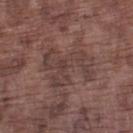Clinical impression: This lesion was catalogued during total-body skin photography and was not selected for biopsy. Image and clinical context: From the left lower leg. The tile uses white-light illumination. The subject is a male aged approximately 75. Longest diameter approximately 6 mm. Cropped from a total-body skin-imaging series; the visible field is about 15 mm.